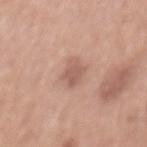lesion_size:
  long_diameter_mm_approx: 3.5
image:
  source: total-body photography crop
  field_of_view_mm: 15
automated_metrics:
  area_mm2_approx: 6.0
  eccentricity: 0.8
  shape_asymmetry: 0.2
  vs_skin_darker_L: 9.0
  vs_skin_contrast_norm: 6.0
  border_irregularity_0_10: 2.0
  color_variation_0_10: 2.0
  peripheral_color_asymmetry: 1.0
  nevus_likeness_0_100: 5
  lesion_detection_confidence_0_100: 100
lighting: white-light
site: mid back
patient:
  sex: male
  age_approx: 70